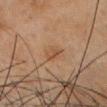  biopsy_status: not biopsied; imaged during a skin examination
  image:
    source: total-body photography crop
    field_of_view_mm: 15
  patient:
    sex: female
    age_approx: 45
  site: head or neck
  lesion_size:
    long_diameter_mm_approx: 2.5
  lighting: cross-polarized